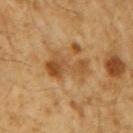Captured during whole-body skin photography for melanoma surveillance; the lesion was not biopsied.
On the right upper arm.
Automated image analysis of the tile measured a mean CIELAB color near L≈46 a*≈19 b*≈37, about 9 CIELAB-L* units darker than the surrounding skin, and a normalized border contrast of about 7. It also reported a nevus-likeness score of about 15/100 and lesion-presence confidence of about 100/100.
A male patient aged 58 to 62.
A 15 mm close-up extracted from a 3D total-body photography capture.